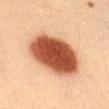<case>
<biopsy_status>not biopsied; imaged during a skin examination</biopsy_status>
<lesion_size>
  <long_diameter_mm_approx>6.5</long_diameter_mm_approx>
</lesion_size>
<patient>
  <sex>male</sex>
  <age_approx>40</age_approx>
</patient>
<lighting>cross-polarized</lighting>
<image>
  <source>total-body photography crop</source>
  <field_of_view_mm>15</field_of_view_mm>
</image>
<site>front of the torso</site>
</case>